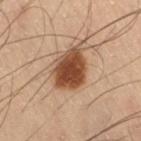biopsy status: total-body-photography surveillance lesion; no biopsy | acquisition: 15 mm crop, total-body photography | anatomic site: the right thigh | patient: male, aged approximately 55 | size: ~4.5 mm (longest diameter) | lighting: cross-polarized illumination.